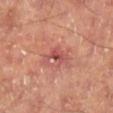follow-up: imaged on a skin check; not biopsied
image: 15 mm crop, total-body photography
patient: male, about 65 years old
location: the right lower leg
automated metrics: a lesion area of about 5 mm², a shape eccentricity near 0.85, and a shape-asymmetry score of about 0.5 (0 = symmetric); an automated nevus-likeness rating near 0 out of 100
lighting: cross-polarized illumination
lesion diameter: about 4 mm